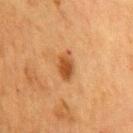Q: Is there a histopathology result?
A: no biopsy performed (imaged during a skin exam)
Q: Lesion location?
A: the front of the torso
Q: What kind of image is this?
A: ~15 mm crop, total-body skin-cancer survey
Q: How was the tile lit?
A: cross-polarized
Q: Patient demographics?
A: female, aged approximately 55
Q: What did automated image analysis measure?
A: an area of roughly 5.5 mm², a shape eccentricity near 0.8, and two-axis asymmetry of about 0.2; a lesion color around L≈43 a*≈22 b*≈36 in CIELAB, roughly 11 lightness units darker than nearby skin, and a normalized border contrast of about 8.5; a classifier nevus-likeness of about 90/100 and a detector confidence of about 100 out of 100 that the crop contains a lesion
Q: How large is the lesion?
A: ≈3.5 mm